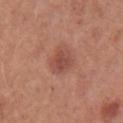Assessment: Captured during whole-body skin photography for melanoma surveillance; the lesion was not biopsied. Context: Imaged with white-light lighting. The recorded lesion diameter is about 3 mm. Cropped from a total-body skin-imaging series; the visible field is about 15 mm. A female patient roughly 45 years of age. The lesion is located on the left upper arm.